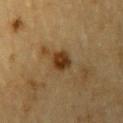Part of a total-body skin-imaging series; this lesion was reviewed on a skin check and was not flagged for biopsy.
This image is a 15 mm lesion crop taken from a total-body photograph.
The lesion is located on the left upper arm.
The patient is a male aged approximately 85 or older.
Captured under cross-polarized illumination.
Approximately 3 mm at its widest.